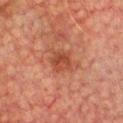  biopsy_status: not biopsied; imaged during a skin examination
  site: chest
  patient:
    sex: male
    age_approx: 75
  image:
    source: total-body photography crop
    field_of_view_mm: 15
  lighting: cross-polarized